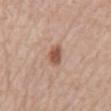The lesion was tiled from a total-body skin photograph and was not biopsied. The tile uses white-light illumination. The lesion's longest dimension is about 3 mm. The subject is a male approximately 80 years of age. A 15 mm close-up extracted from a 3D total-body photography capture. The lesion is located on the back.